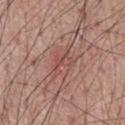Q: Is there a histopathology result?
A: imaged on a skin check; not biopsied